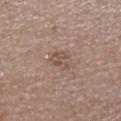notes = catalogued during a skin exam; not biopsied | subject = female, aged 68–72 | automated metrics = a footprint of about 4.5 mm² and a shape eccentricity near 0.45 | acquisition = ~15 mm tile from a whole-body skin photo | lesion diameter = ≈2.5 mm | body site = the left lower leg.